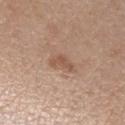Clinical impression:
No biopsy was performed on this lesion — it was imaged during a full skin examination and was not determined to be concerning.
Image and clinical context:
Approximately 3 mm at its widest. A male subject aged 58–62. A 15 mm close-up tile from a total-body photography series done for melanoma screening. On the arm. The lesion-visualizer software estimated a color-variation rating of about 1.5/10 and peripheral color asymmetry of about 0.5. And it measured a nevus-likeness score of about 0/100 and lesion-presence confidence of about 100/100.